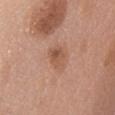From the abdomen. A 15 mm close-up tile from a total-body photography series done for melanoma screening. The recorded lesion diameter is about 3.5 mm. Captured under white-light illumination. A female subject, aged 58 to 62.